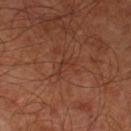Clinical impression: No biopsy was performed on this lesion — it was imaged during a full skin examination and was not determined to be concerning. Image and clinical context: The total-body-photography lesion software estimated an area of roughly 3 mm² and two-axis asymmetry of about 0.5. The software also gave a border-irregularity index near 6.5/10, a color-variation rating of about 0/10, and radial color variation of about 0. The lesion's longest dimension is about 3 mm. The tile uses cross-polarized illumination. The lesion is located on the left lower leg. The patient is approximately 65 years of age. A 15 mm close-up tile from a total-body photography series done for melanoma screening.